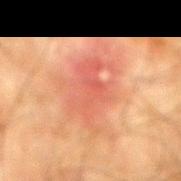Part of a total-body skin-imaging series; this lesion was reviewed on a skin check and was not flagged for biopsy. A lesion tile, about 15 mm wide, cut from a 3D total-body photograph. A male patient, about 65 years old. The lesion is on the mid back. This is a cross-polarized tile.